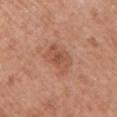Impression: Part of a total-body skin-imaging series; this lesion was reviewed on a skin check and was not flagged for biopsy. Context: A female patient, roughly 60 years of age. A region of skin cropped from a whole-body photographic capture, roughly 15 mm wide. The lesion-visualizer software estimated roughly 8 lightness units darker than nearby skin and a lesion-to-skin contrast of about 6 (normalized; higher = more distinct). The software also gave a border-irregularity index near 3/10 and a color-variation rating of about 3/10. And it measured a detector confidence of about 100 out of 100 that the crop contains a lesion. Located on the left upper arm.